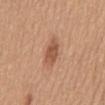| feature | finding |
|---|---|
| workup | imaged on a skin check; not biopsied |
| automated metrics | an average lesion color of about L≈53 a*≈23 b*≈32 (CIELAB) |
| image source | total-body-photography crop, ~15 mm field of view |
| patient | female, aged around 55 |
| site | the mid back |
| lesion diameter | ~3.5 mm (longest diameter) |